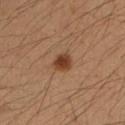Imaged during a routine full-body skin examination; the lesion was not biopsied and no histopathology is available. Longest diameter approximately 2.5 mm. A close-up tile cropped from a whole-body skin photograph, about 15 mm across. A female subject aged around 35. Captured under cross-polarized illumination. On the arm.